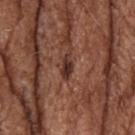follow-up — no biopsy performed (imaged during a skin exam)
body site — the head or neck
lesion diameter — ≈3 mm
image source — ~15 mm tile from a whole-body skin photo
lighting — white-light
patient — male, aged around 75